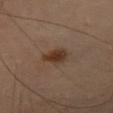Recorded during total-body skin imaging; not selected for excision or biopsy. A lesion tile, about 15 mm wide, cut from a 3D total-body photograph. A male subject, about 65 years old. On the right thigh. Captured under cross-polarized illumination. An algorithmic analysis of the crop reported a lesion area of about 6 mm², a shape eccentricity near 0.65, and two-axis asymmetry of about 0.25. And it measured a border-irregularity rating of about 2.5/10 and a peripheral color-asymmetry measure near 1.